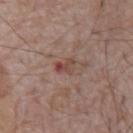No biopsy was performed on this lesion — it was imaged during a full skin examination and was not determined to be concerning. The lesion is on the abdomen. Measured at roughly 2.5 mm in maximum diameter. Automated image analysis of the tile measured an area of roughly 3.5 mm², an eccentricity of roughly 0.75, and a shape-asymmetry score of about 0.25 (0 = symmetric). And it measured border irregularity of about 2.5 on a 0–10 scale and internal color variation of about 6.5 on a 0–10 scale. The analysis additionally found a nevus-likeness score of about 0/100 and lesion-presence confidence of about 100/100. A 15 mm close-up extracted from a 3D total-body photography capture. The patient is a male approximately 70 years of age.